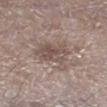notes: total-body-photography surveillance lesion; no biopsy | site: the left lower leg | automated metrics: a lesion area of about 12 mm² and a symmetry-axis asymmetry near 0.35 | lighting: white-light illumination | subject: male, approximately 65 years of age | image: 15 mm crop, total-body photography.